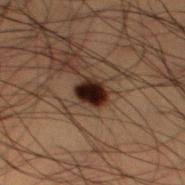Assessment:
The lesion was photographed on a routine skin check and not biopsied; there is no pathology result.
Image and clinical context:
A 15 mm crop from a total-body photograph taken for skin-cancer surveillance. The recorded lesion diameter is about 3 mm. From the leg. The tile uses cross-polarized illumination. Automated image analysis of the tile measured an area of roughly 6 mm², an outline eccentricity of about 0.55 (0 = round, 1 = elongated), and a shape-asymmetry score of about 0.2 (0 = symmetric). It also reported a border-irregularity index near 2/10 and a peripheral color-asymmetry measure near 1.5. It also reported a nevus-likeness score of about 90/100. The subject is a male aged 48–52.